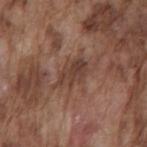biopsy status: catalogued during a skin exam; not biopsied | illumination: white-light illumination | patient: male, roughly 75 years of age | location: the mid back | automated metrics: a lesion area of about 7 mm², an eccentricity of roughly 0.8, and two-axis asymmetry of about 0.3; an automated nevus-likeness rating near 0 out of 100 and a detector confidence of about 95 out of 100 that the crop contains a lesion | lesion diameter: about 4 mm | acquisition: ~15 mm tile from a whole-body skin photo.